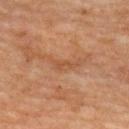notes = catalogued during a skin exam; not biopsied | image source = 15 mm crop, total-body photography | lesion size = about 3 mm | lighting = cross-polarized | anatomic site = the upper back | patient = female, approximately 70 years of age.